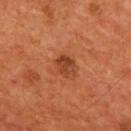  biopsy_status: not biopsied; imaged during a skin examination
  image:
    source: total-body photography crop
    field_of_view_mm: 15
  lesion_size:
    long_diameter_mm_approx: 2.5
  lighting: cross-polarized
  patient:
    sex: male
    age_approx: 55
  site: chest
  automated_metrics:
    area_mm2_approx: 4.5
    eccentricity: 0.6
    shape_asymmetry: 0.15
    cielab_L: 39
    cielab_a: 26
    cielab_b: 34
    vs_skin_darker_L: 9.0
    vs_skin_contrast_norm: 7.5
    color_variation_0_10: 3.5
    peripheral_color_asymmetry: 1.0
    nevus_likeness_0_100: 10
    lesion_detection_confidence_0_100: 100